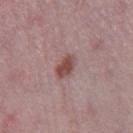A female subject, in their mid- to late 40s. Approximately 3 mm at its widest. A region of skin cropped from a whole-body photographic capture, roughly 15 mm wide. On the right thigh. An algorithmic analysis of the crop reported lesion-presence confidence of about 100/100.